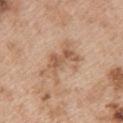notes: catalogued during a skin exam; not biopsied | subject: female, roughly 45 years of age | lighting: white-light | body site: the upper back | lesion size: ~6 mm (longest diameter) | image source: 15 mm crop, total-body photography | automated metrics: an average lesion color of about L≈59 a*≈19 b*≈32 (CIELAB), roughly 9 lightness units darker than nearby skin, and a lesion-to-skin contrast of about 6 (normalized; higher = more distinct); a within-lesion color-variation index near 5/10 and peripheral color asymmetry of about 2.